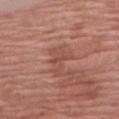{"biopsy_status": "not biopsied; imaged during a skin examination", "image": {"source": "total-body photography crop", "field_of_view_mm": 15}, "lesion_size": {"long_diameter_mm_approx": 3.5}, "site": "right upper arm", "lighting": "white-light", "automated_metrics": {"shape_asymmetry": 0.5, "border_irregularity_0_10": 6.0, "peripheral_color_asymmetry": 1.0}, "patient": {"sex": "female", "age_approx": 70}}